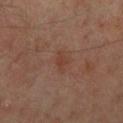Q: Is there a histopathology result?
A: catalogued during a skin exam; not biopsied
Q: How was this image acquired?
A: 15 mm crop, total-body photography
Q: Automated lesion metrics?
A: an area of roughly 3 mm²; a within-lesion color-variation index near 1/10 and a peripheral color-asymmetry measure near 0
Q: What is the lesion's diameter?
A: ≈2.5 mm
Q: Patient demographics?
A: male, aged 48–52
Q: Where on the body is the lesion?
A: the left leg